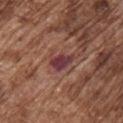Assessment: Captured during whole-body skin photography for melanoma surveillance; the lesion was not biopsied.